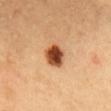Q: Was this lesion biopsied?
A: total-body-photography surveillance lesion; no biopsy
Q: Lesion size?
A: ~3 mm (longest diameter)
Q: Lesion location?
A: the head or neck
Q: What are the patient's age and sex?
A: female, approximately 35 years of age
Q: What did automated image analysis measure?
A: a footprint of about 7 mm², an outline eccentricity of about 0.6 (0 = round, 1 = elongated), and a symmetry-axis asymmetry near 0.15; an average lesion color of about L≈48 a*≈27 b*≈38 (CIELAB) and roughly 20 lightness units darker than nearby skin; a classifier nevus-likeness of about 100/100 and lesion-presence confidence of about 100/100
Q: What is the imaging modality?
A: 15 mm crop, total-body photography